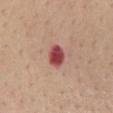Part of a total-body skin-imaging series; this lesion was reviewed on a skin check and was not flagged for biopsy.